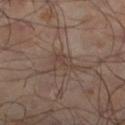<lesion>
<biopsy_status>not biopsied; imaged during a skin examination</biopsy_status>
<patient>
  <sex>male</sex>
  <age_approx>65</age_approx>
</patient>
<image>
  <source>total-body photography crop</source>
  <field_of_view_mm>15</field_of_view_mm>
</image>
<site>left lower leg</site>
<lighting>cross-polarized</lighting>
<lesion_size>
  <long_diameter_mm_approx>3.0</long_diameter_mm_approx>
</lesion_size>
</lesion>